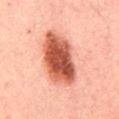Recorded during total-body skin imaging; not selected for excision or biopsy. On the back. A close-up tile cropped from a whole-body skin photograph, about 15 mm across. A male patient roughly 30 years of age.